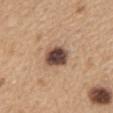Q: Is there a histopathology result?
A: no biopsy performed (imaged during a skin exam)
Q: Illumination type?
A: white-light
Q: Lesion size?
A: about 3.5 mm
Q: What kind of image is this?
A: ~15 mm tile from a whole-body skin photo
Q: What is the anatomic site?
A: the upper back
Q: Patient demographics?
A: female, in their 60s
Q: What did automated image analysis measure?
A: a lesion color around L≈46 a*≈17 b*≈25 in CIELAB and about 20 CIELAB-L* units darker than the surrounding skin; a border-irregularity rating of about 1/10, a within-lesion color-variation index near 5.5/10, and peripheral color asymmetry of about 1.5